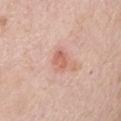Q: Was a biopsy performed?
A: catalogued during a skin exam; not biopsied
Q: What is the anatomic site?
A: the abdomen
Q: What lighting was used for the tile?
A: white-light
Q: Patient demographics?
A: female, aged approximately 75
Q: What kind of image is this?
A: ~15 mm crop, total-body skin-cancer survey